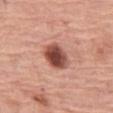Q: Is there a histopathology result?
A: total-body-photography surveillance lesion; no biopsy
Q: How was the tile lit?
A: white-light
Q: Lesion location?
A: the left thigh
Q: What is the imaging modality?
A: 15 mm crop, total-body photography
Q: What is the lesion's diameter?
A: ≈4 mm
Q: Who is the patient?
A: female, aged around 65